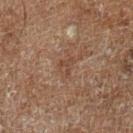Notes:
• biopsy status: total-body-photography surveillance lesion; no biopsy
• site: the left lower leg
• TBP lesion metrics: a lesion area of about 4 mm² and an outline eccentricity of about 0.7 (0 = round, 1 = elongated); border irregularity of about 4 on a 0–10 scale, a color-variation rating of about 1.5/10, and a peripheral color-asymmetry measure near 0.5
• tile lighting: cross-polarized illumination
• patient: male, approximately 60 years of age
• imaging modality: 15 mm crop, total-body photography